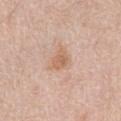Clinical impression: Imaged during a routine full-body skin examination; the lesion was not biopsied and no histopathology is available. Background: On the front of the torso. Longest diameter approximately 3 mm. A roughly 15 mm field-of-view crop from a total-body skin photograph. A male subject, aged 78 to 82. The tile uses white-light illumination.